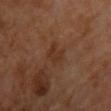follow-up = total-body-photography surveillance lesion; no biopsy | site = the front of the torso | subject = male, in their 60s | imaging modality = 15 mm crop, total-body photography.